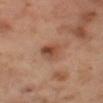biopsy_status: not biopsied; imaged during a skin examination
site: left thigh
lighting: cross-polarized
image:
  source: total-body photography crop
  field_of_view_mm: 15
lesion_size:
  long_diameter_mm_approx: 3.0
patient:
  sex: female
  age_approx: 55